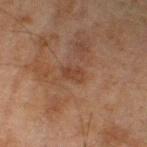patient — male, approximately 45 years of age
anatomic site — the left lower leg
imaging modality — 15 mm crop, total-body photography